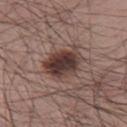<case>
<biopsy_status>not biopsied; imaged during a skin examination</biopsy_status>
<patient>
  <sex>male</sex>
  <age_approx>55</age_approx>
</patient>
<image>
  <source>total-body photography crop</source>
  <field_of_view_mm>15</field_of_view_mm>
</image>
<site>right thigh</site>
<lighting>white-light</lighting>
<lesion_size>
  <long_diameter_mm_approx>5.0</long_diameter_mm_approx>
</lesion_size>
<automated_metrics>
  <area_mm2_approx>13.0</area_mm2_approx>
  <eccentricity>0.75</eccentricity>
  <shape_asymmetry>0.15</shape_asymmetry>
  <nevus_likeness_0_100>85</nevus_likeness_0_100>
  <lesion_detection_confidence_0_100>100</lesion_detection_confidence_0_100>
</automated_metrics>
</case>